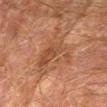Q: Was a biopsy performed?
A: catalogued during a skin exam; not biopsied
Q: Lesion size?
A: ≈6.5 mm
Q: What is the imaging modality?
A: 15 mm crop, total-body photography
Q: Patient demographics?
A: male, approximately 75 years of age
Q: Lesion location?
A: the left forearm